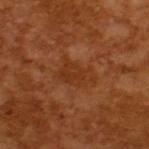workup = no biopsy performed (imaged during a skin exam) | image source = 15 mm crop, total-body photography | patient = male, aged 63–67 | diameter = about 4.5 mm | tile lighting = cross-polarized illumination | TBP lesion metrics = a lesion color around L≈34 a*≈25 b*≈35 in CIELAB, a lesion–skin lightness drop of about 5, and a normalized lesion–skin contrast near 5; border irregularity of about 4 on a 0–10 scale, a color-variation rating of about 2.5/10, and a peripheral color-asymmetry measure near 1; a nevus-likeness score of about 0/100 and lesion-presence confidence of about 100/100.